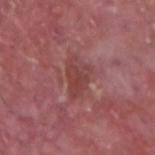Imaged during a routine full-body skin examination; the lesion was not biopsied and no histopathology is available. The lesion is located on the left forearm. A region of skin cropped from a whole-body photographic capture, roughly 15 mm wide. A male patient aged around 40.